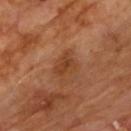Clinical impression:
Part of a total-body skin-imaging series; this lesion was reviewed on a skin check and was not flagged for biopsy.
Context:
A male subject aged around 60. Measured at roughly 3 mm in maximum diameter. A region of skin cropped from a whole-body photographic capture, roughly 15 mm wide. The total-body-photography lesion software estimated border irregularity of about 3.5 on a 0–10 scale, internal color variation of about 2.5 on a 0–10 scale, and a peripheral color-asymmetry measure near 1. And it measured a classifier nevus-likeness of about 10/100 and a lesion-detection confidence of about 100/100. This is a cross-polarized tile. The lesion is located on the upper back.